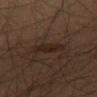{"biopsy_status": "not biopsied; imaged during a skin examination"}